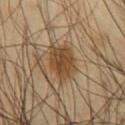Notes:
- image — ~15 mm tile from a whole-body skin photo
- illumination — cross-polarized
- lesion diameter — ≈5 mm
- subject — male, about 40 years old
- location — the chest
- automated metrics — a nevus-likeness score of about 90/100